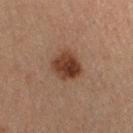Imaged during a routine full-body skin examination; the lesion was not biopsied and no histopathology is available.
A 15 mm close-up extracted from a 3D total-body photography capture.
The lesion-visualizer software estimated an average lesion color of about L≈30 a*≈18 b*≈24 (CIELAB), roughly 11 lightness units darker than nearby skin, and a normalized lesion–skin contrast near 11. The software also gave a border-irregularity rating of about 1.5/10 and peripheral color asymmetry of about 2. And it measured an automated nevus-likeness rating near 100 out of 100 and a detector confidence of about 100 out of 100 that the crop contains a lesion.
Located on the right thigh.
This is a cross-polarized tile.
A female patient, about 60 years old.
The recorded lesion diameter is about 3.5 mm.